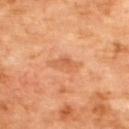Cropped from a whole-body photographic skin survey; the tile spans about 15 mm. The subject is a male about 70 years old. On the upper back.